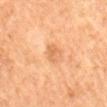Assessment: No biopsy was performed on this lesion — it was imaged during a full skin examination and was not determined to be concerning. Acquisition and patient details: The lesion's longest dimension is about 2.5 mm. The subject is a female roughly 60 years of age. The total-body-photography lesion software estimated an average lesion color of about L≈54 a*≈21 b*≈34 (CIELAB) and roughly 7 lightness units darker than nearby skin. It also reported border irregularity of about 2.5 on a 0–10 scale, a color-variation rating of about 3/10, and radial color variation of about 1. Imaged with cross-polarized lighting. A roughly 15 mm field-of-view crop from a total-body skin photograph. On the back.